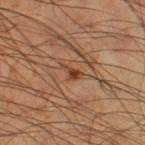Clinical impression:
Recorded during total-body skin imaging; not selected for excision or biopsy.
Background:
Longest diameter approximately 2.5 mm. Located on the right thigh. A region of skin cropped from a whole-body photographic capture, roughly 15 mm wide. Automated tile analysis of the lesion measured a lesion area of about 2.5 mm² and an outline eccentricity of about 0.85 (0 = round, 1 = elongated). And it measured an average lesion color of about L≈42 a*≈22 b*≈32 (CIELAB), a lesion–skin lightness drop of about 10, and a lesion-to-skin contrast of about 8 (normalized; higher = more distinct). It also reported border irregularity of about 5 on a 0–10 scale, a color-variation rating of about 1.5/10, and peripheral color asymmetry of about 0.5. A male patient in their 60s.